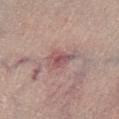Clinical impression: Recorded during total-body skin imaging; not selected for excision or biopsy.